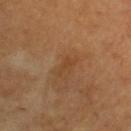notes: no biopsy performed (imaged during a skin exam)
TBP lesion metrics: a lesion area of about 2.5 mm² and an outline eccentricity of about 0.9 (0 = round, 1 = elongated); a lesion-to-skin contrast of about 4.5 (normalized; higher = more distinct); a border-irregularity index near 4/10, internal color variation of about 0 on a 0–10 scale, and a peripheral color-asymmetry measure near 0; a classifier nevus-likeness of about 0/100
lesion size: ≈2.5 mm
patient: aged 58–62
illumination: cross-polarized
site: the upper back
imaging modality: ~15 mm crop, total-body skin-cancer survey